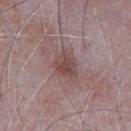Clinical summary:
A 15 mm crop from a total-body photograph taken for skin-cancer surveillance. A male subject, aged around 65. Captured under white-light illumination. Longest diameter approximately 4 mm. The lesion is located on the chest.